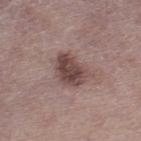Findings:
* workup: no biopsy performed (imaged during a skin exam)
* subject: male, aged 73–77
* lesion size: ~4.5 mm (longest diameter)
* location: the right lower leg
* image: total-body-photography crop, ~15 mm field of view